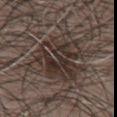Assessment: Captured during whole-body skin photography for melanoma surveillance; the lesion was not biopsied.